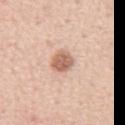- biopsy status — total-body-photography surveillance lesion; no biopsy
- image source — 15 mm crop, total-body photography
- site — the front of the torso
- diameter — about 3 mm
- patient — male, aged 53 to 57